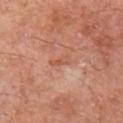{
  "biopsy_status": "not biopsied; imaged during a skin examination",
  "image": {
    "source": "total-body photography crop",
    "field_of_view_mm": 15
  },
  "lighting": "cross-polarized",
  "lesion_size": {
    "long_diameter_mm_approx": 2.5
  },
  "patient": {
    "sex": "male",
    "age_approx": 70
  },
  "site": "left thigh"
}